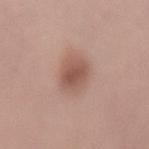{"biopsy_status": "not biopsied; imaged during a skin examination", "site": "lower back", "patient": {"sex": "male", "age_approx": 30}, "image": {"source": "total-body photography crop", "field_of_view_mm": 15}}